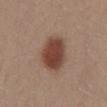Notes:
– patient — female, aged approximately 30
– acquisition — 15 mm crop, total-body photography
– location — the chest
– illumination — white-light
– lesion size — ≈4.5 mm